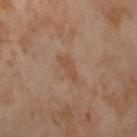illumination: cross-polarized; imaging modality: ~15 mm tile from a whole-body skin photo; lesion diameter: ≈3.5 mm; subject: female, in their mid- to late 50s; anatomic site: the right thigh.